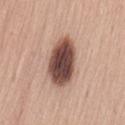• workup — no biopsy performed (imaged during a skin exam)
• image — ~15 mm crop, total-body skin-cancer survey
• lesion size — ~6 mm (longest diameter)
• automated lesion analysis — roughly 22 lightness units darker than nearby skin and a lesion-to-skin contrast of about 15 (normalized; higher = more distinct)
• patient — female, roughly 30 years of age
• lighting — white-light
• location — the lower back